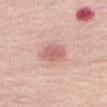Recorded during total-body skin imaging; not selected for excision or biopsy. The tile uses white-light illumination. Located on the front of the torso. A female patient about 50 years old. The lesion's longest dimension is about 3.5 mm. A 15 mm close-up extracted from a 3D total-body photography capture.